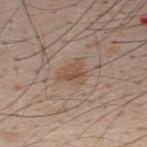Impression: This lesion was catalogued during total-body skin photography and was not selected for biopsy. Background: A region of skin cropped from a whole-body photographic capture, roughly 15 mm wide. This is a white-light tile. The lesion is located on the upper back. A male patient, aged 33–37. Automated image analysis of the tile measured a shape eccentricity near 0.6. The software also gave a classifier nevus-likeness of about 5/100 and lesion-presence confidence of about 100/100. Longest diameter approximately 3 mm.